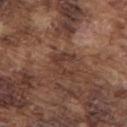This lesion was catalogued during total-body skin photography and was not selected for biopsy. A 15 mm close-up extracted from a 3D total-body photography capture. About 3 mm across. Imaged with white-light lighting. A male patient, aged 73 to 77. On the upper back.